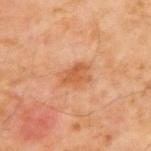| key | value |
|---|---|
| subject | male, approximately 60 years of age |
| body site | the upper back |
| illumination | cross-polarized illumination |
| size | ~3.5 mm (longest diameter) |
| acquisition | ~15 mm crop, total-body skin-cancer survey |